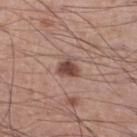biopsy status: total-body-photography surveillance lesion; no biopsy.